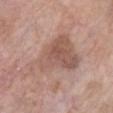Part of a total-body skin-imaging series; this lesion was reviewed on a skin check and was not flagged for biopsy.
Captured under white-light illumination.
A close-up tile cropped from a whole-body skin photograph, about 15 mm across.
A male subject, aged around 60.
On the chest.
The total-body-photography lesion software estimated border irregularity of about 5.5 on a 0–10 scale and a peripheral color-asymmetry measure near 1.5.
About 8 mm across.